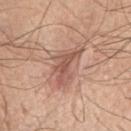Findings:
- subject · male, aged 63–67
- illumination · white-light
- acquisition · 15 mm crop, total-body photography
- anatomic site · the upper back